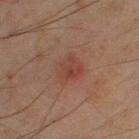{
  "biopsy_status": "not biopsied; imaged during a skin examination",
  "automated_metrics": {
    "cielab_L": 34,
    "cielab_a": 19,
    "cielab_b": 22,
    "vs_skin_darker_L": 5.0,
    "vs_skin_contrast_norm": 5.0
  },
  "patient": {
    "sex": "male",
    "age_approx": 45
  },
  "image": {
    "source": "total-body photography crop",
    "field_of_view_mm": 15
  },
  "site": "left thigh"
}